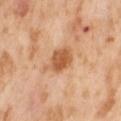{"biopsy_status": "not biopsied; imaged during a skin examination", "lighting": "cross-polarized", "patient": {"sex": "female", "age_approx": 55}, "automated_metrics": {"area_mm2_approx": 8.0}, "lesion_size": {"long_diameter_mm_approx": 4.5}, "site": "abdomen", "image": {"source": "total-body photography crop", "field_of_view_mm": 15}}